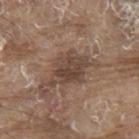<lesion>
  <biopsy_status>not biopsied; imaged during a skin examination</biopsy_status>
  <site>upper back</site>
  <lesion_size>
    <long_diameter_mm_approx>5.5</long_diameter_mm_approx>
  </lesion_size>
  <automated_metrics>
    <area_mm2_approx>12.0</area_mm2_approx>
    <eccentricity>0.75</eccentricity>
    <shape_asymmetry>0.25</shape_asymmetry>
    <color_variation_0_10>4.0</color_variation_0_10>
    <peripheral_color_asymmetry>1.5</peripheral_color_asymmetry>
  </automated_metrics>
  <image>
    <source>total-body photography crop</source>
    <field_of_view_mm>15</field_of_view_mm>
  </image>
  <patient>
    <sex>male</sex>
    <age_approx>80</age_approx>
  </patient>
</lesion>